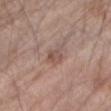biopsy status=catalogued during a skin exam; not biopsied
illumination=white-light illumination
patient=male, aged 78 to 82
acquisition=~15 mm tile from a whole-body skin photo
location=the right forearm
diameter=about 2.5 mm
image-analysis metrics=about 8 CIELAB-L* units darker than the surrounding skin